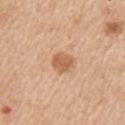Findings:
• biopsy status · catalogued during a skin exam; not biopsied
• acquisition · ~15 mm crop, total-body skin-cancer survey
• subject · male, roughly 60 years of age
• site · the left upper arm